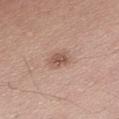Clinical impression:
The lesion was photographed on a routine skin check and not biopsied; there is no pathology result.
Clinical summary:
The total-body-photography lesion software estimated a border-irregularity rating of about 2/10 and a within-lesion color-variation index near 3.5/10. Located on the abdomen. This image is a 15 mm lesion crop taken from a total-body photograph. A male patient, aged 53 to 57. Longest diameter approximately 3 mm.